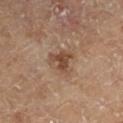{"biopsy_status": "not biopsied; imaged during a skin examination", "site": "right lower leg", "lesion_size": {"long_diameter_mm_approx": 3.0}, "patient": {"sex": "male", "age_approx": 65}, "lighting": "cross-polarized", "automated_metrics": {"cielab_L": 47, "cielab_a": 19, "cielab_b": 29, "vs_skin_darker_L": 10.0}, "image": {"source": "total-body photography crop", "field_of_view_mm": 15}}